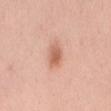{"biopsy_status": "not biopsied; imaged during a skin examination", "image": {"source": "total-body photography crop", "field_of_view_mm": 15}, "patient": {"sex": "female", "age_approx": 35}, "site": "mid back"}